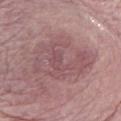Recorded during total-body skin imaging; not selected for excision or biopsy. About 7.5 mm across. A female subject, aged around 70. A 15 mm close-up tile from a total-body photography series done for melanoma screening. From the front of the torso.